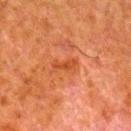<lesion>
  <biopsy_status>not biopsied; imaged during a skin examination</biopsy_status>
  <image>
    <source>total-body photography crop</source>
    <field_of_view_mm>15</field_of_view_mm>
  </image>
  <site>leg</site>
  <patient>
    <sex>male</sex>
    <age_approx>80</age_approx>
  </patient>
</lesion>